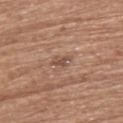workup — catalogued during a skin exam; not biopsied | lighting — white-light illumination | subject — female, aged approximately 65 | imaging modality — total-body-photography crop, ~15 mm field of view | lesion size — ~2.5 mm (longest diameter) | anatomic site — the upper back.